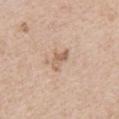Recorded during total-body skin imaging; not selected for excision or biopsy. The lesion is located on the chest. Measured at roughly 3 mm in maximum diameter. Automated image analysis of the tile measured an eccentricity of roughly 0.85 and two-axis asymmetry of about 0.4. It also reported a mean CIELAB color near L≈61 a*≈18 b*≈31, roughly 10 lightness units darker than nearby skin, and a lesion-to-skin contrast of about 6.5 (normalized; higher = more distinct). And it measured an automated nevus-likeness rating near 5 out of 100 and a lesion-detection confidence of about 100/100. A female patient in their 40s. Cropped from a total-body skin-imaging series; the visible field is about 15 mm.